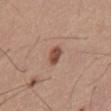The lesion was tiled from a total-body skin photograph and was not biopsied.
Located on the abdomen.
The recorded lesion diameter is about 2.5 mm.
The total-body-photography lesion software estimated a lesion area of about 4 mm², an outline eccentricity of about 0.75 (0 = round, 1 = elongated), and a symmetry-axis asymmetry near 0.25. The software also gave a mean CIELAB color near L≈49 a*≈22 b*≈28, roughly 13 lightness units darker than nearby skin, and a normalized lesion–skin contrast near 9.5.
The patient is a male aged 53–57.
A region of skin cropped from a whole-body photographic capture, roughly 15 mm wide.
The tile uses white-light illumination.